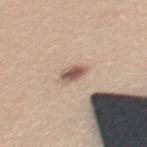Recorded during total-body skin imaging; not selected for excision or biopsy.